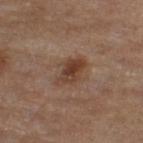biopsy status: imaged on a skin check; not biopsied
patient: male, aged 83 or over
lesion diameter: ≈3.5 mm
location: the right thigh
imaging modality: 15 mm crop, total-body photography
illumination: cross-polarized